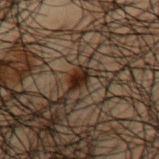Case summary:
* follow-up · imaged on a skin check; not biopsied
* image source · total-body-photography crop, ~15 mm field of view
* size · about 2.5 mm
* subject · male, roughly 50 years of age
* body site · the arm